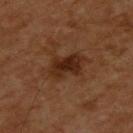On the upper back. Approximately 4.5 mm at its widest. A male patient, aged around 60. A lesion tile, about 15 mm wide, cut from a 3D total-body photograph. Imaged with cross-polarized lighting.